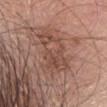Case summary:
• notes: imaged on a skin check; not biopsied
• site: the head or neck
• patient: female, aged around 65
• TBP lesion metrics: a mean CIELAB color near L≈48 a*≈21 b*≈26, a lesion–skin lightness drop of about 7, and a lesion-to-skin contrast of about 5.5 (normalized; higher = more distinct)
• imaging modality: ~15 mm crop, total-body skin-cancer survey
• lesion size: ≈5.5 mm